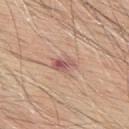| feature | finding |
|---|---|
| lesion size | ≈3 mm |
| subject | male, aged 63–67 |
| image | total-body-photography crop, ~15 mm field of view |
| anatomic site | the upper back |
| illumination | white-light illumination |